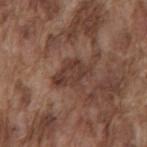The lesion was photographed on a routine skin check and not biopsied; there is no pathology result. An algorithmic analysis of the crop reported a lesion color around L≈38 a*≈19 b*≈24 in CIELAB and a normalized lesion–skin contrast near 7.5. It also reported a classifier nevus-likeness of about 10/100. A roughly 15 mm field-of-view crop from a total-body skin photograph. The lesion is located on the mid back. Longest diameter approximately 4 mm. A male patient aged 73–77.